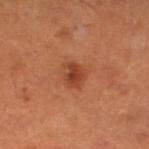<record>
  <lighting>cross-polarized</lighting>
  <image>
    <source>total-body photography crop</source>
    <field_of_view_mm>15</field_of_view_mm>
  </image>
  <site>left lower leg</site>
  <patient>
    <sex>male</sex>
    <age_approx>50</age_approx>
  </patient>
  <lesion_size>
    <long_diameter_mm_approx>3.0</long_diameter_mm_approx>
  </lesion_size>
</record>